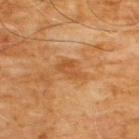{
  "biopsy_status": "not biopsied; imaged during a skin examination",
  "patient": {
    "sex": "male",
    "age_approx": 60
  },
  "lighting": "cross-polarized",
  "automated_metrics": {
    "eccentricity": 0.8,
    "shape_asymmetry": 0.35,
    "border_irregularity_0_10": 3.5,
    "peripheral_color_asymmetry": 0.5,
    "nevus_likeness_0_100": 0
  },
  "lesion_size": {
    "long_diameter_mm_approx": 3.5
  },
  "image": {
    "source": "total-body photography crop",
    "field_of_view_mm": 15
  },
  "site": "chest"
}